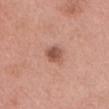workup = no biopsy performed (imaged during a skin exam); subject = female, roughly 20 years of age; site = the head or neck; imaging modality = 15 mm crop, total-body photography; size = ~2.5 mm (longest diameter); tile lighting = white-light illumination.